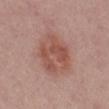biopsy status = imaged on a skin check; not biopsied | imaging modality = total-body-photography crop, ~15 mm field of view | subject = female, aged 38–42 | location = the mid back | diameter = about 6 mm | automated lesion analysis = a border-irregularity index near 2/10, a within-lesion color-variation index near 4.5/10, and radial color variation of about 1.5; a lesion-detection confidence of about 100/100.